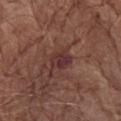biopsy status — total-body-photography surveillance lesion; no biopsy | TBP lesion metrics — a mean CIELAB color near L≈33 a*≈22 b*≈20, a lesion–skin lightness drop of about 8, and a normalized lesion–skin contrast near 8 | imaging modality — ~15 mm tile from a whole-body skin photo | subject — male, approximately 75 years of age | size — ≈3 mm | illumination — white-light illumination | site — the chest.